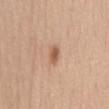biopsy status — catalogued during a skin exam; not biopsied | automated lesion analysis — an area of roughly 3 mm², an outline eccentricity of about 0.9 (0 = round, 1 = elongated), and a shape-asymmetry score of about 0.3 (0 = symmetric); an average lesion color of about L≈58 a*≈22 b*≈33 (CIELAB), about 11 CIELAB-L* units darker than the surrounding skin, and a normalized lesion–skin contrast near 8; a classifier nevus-likeness of about 95/100 and lesion-presence confidence of about 100/100 | lighting — white-light illumination | location — the mid back | lesion size — ~3 mm (longest diameter) | image source — ~15 mm crop, total-body skin-cancer survey | patient — female, approximately 35 years of age.